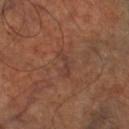* biopsy status: catalogued during a skin exam; not biopsied
* TBP lesion metrics: a mean CIELAB color near L≈37 a*≈20 b*≈25, roughly 5 lightness units darker than nearby skin, and a lesion-to-skin contrast of about 5 (normalized; higher = more distinct)
* illumination: cross-polarized illumination
* lesion size: about 3.5 mm
* anatomic site: the right thigh
* image: 15 mm crop, total-body photography
* subject: aged approximately 65